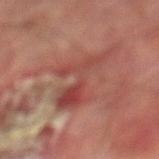Notes:
* notes — total-body-photography surveillance lesion; no biopsy
* subject — male, about 75 years old
* site — the leg
* image-analysis metrics — an area of roughly 15 mm², a shape eccentricity near 0.75, and two-axis asymmetry of about 0.6
* lighting — cross-polarized illumination
* acquisition — 15 mm crop, total-body photography
* lesion size — ≈5.5 mm